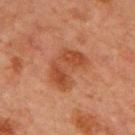The lesion was photographed on a routine skin check and not biopsied; there is no pathology result. Captured under cross-polarized illumination. This image is a 15 mm lesion crop taken from a total-body photograph. A male subject in their mid-60s. On the front of the torso. The lesion-visualizer software estimated a border-irregularity rating of about 3.5/10, internal color variation of about 4 on a 0–10 scale, and a peripheral color-asymmetry measure near 1. It also reported a nevus-likeness score of about 20/100.